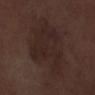Assessment:
No biopsy was performed on this lesion — it was imaged during a full skin examination and was not determined to be concerning.
Image and clinical context:
The patient is a male in their 70s. Automated tile analysis of the lesion measured a footprint of about 35 mm², an eccentricity of roughly 0.75, and a symmetry-axis asymmetry near 0.3. It also reported an average lesion color of about L≈24 a*≈14 b*≈17 (CIELAB), a lesion–skin lightness drop of about 5, and a normalized border contrast of about 6. And it measured a classifier nevus-likeness of about 0/100. A 15 mm close-up extracted from a 3D total-body photography capture. From the right lower leg. About 9 mm across.